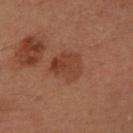Impression:
Recorded during total-body skin imaging; not selected for excision or biopsy.
Clinical summary:
The patient is a female aged around 60. Measured at roughly 4 mm in maximum diameter. The tile uses cross-polarized illumination. The lesion is located on the left forearm. A lesion tile, about 15 mm wide, cut from a 3D total-body photograph.